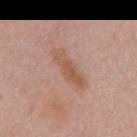Q: Is there a histopathology result?
A: no biopsy performed (imaged during a skin exam)
Q: Where on the body is the lesion?
A: the mid back
Q: Who is the patient?
A: male, aged 53 to 57
Q: What is the imaging modality?
A: ~15 mm crop, total-body skin-cancer survey
Q: What did automated image analysis measure?
A: a lesion color around L≈56 a*≈21 b*≈30 in CIELAB and roughly 8 lightness units darker than nearby skin; border irregularity of about 3.5 on a 0–10 scale, a within-lesion color-variation index near 3.5/10, and a peripheral color-asymmetry measure near 1.5
Q: What lighting was used for the tile?
A: white-light illumination